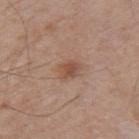follow-up = no biopsy performed (imaged during a skin exam)
image = ~15 mm tile from a whole-body skin photo
patient = male, in their 70s
lesion size = ~3 mm (longest diameter)
location = the upper back
lighting = white-light